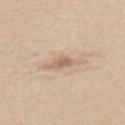<lesion>
  <biopsy_status>not biopsied; imaged during a skin examination</biopsy_status>
  <patient>
    <sex>female</sex>
    <age_approx>30</age_approx>
  </patient>
  <image>
    <source>total-body photography crop</source>
    <field_of_view_mm>15</field_of_view_mm>
  </image>
  <site>mid back</site>
</lesion>